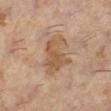<record>
  <biopsy_status>not biopsied; imaged during a skin examination</biopsy_status>
  <lesion_size>
    <long_diameter_mm_approx>5.5</long_diameter_mm_approx>
  </lesion_size>
  <site>left lower leg</site>
  <automated_metrics>
    <area_mm2_approx>13.0</area_mm2_approx>
    <eccentricity>0.8</eccentricity>
    <shape_asymmetry>0.4</shape_asymmetry>
    <color_variation_0_10>2.5</color_variation_0_10>
    <peripheral_color_asymmetry>1.0</peripheral_color_asymmetry>
  </automated_metrics>
  <lighting>cross-polarized</lighting>
  <patient>
    <sex>female</sex>
    <age_approx>60</age_approx>
  </patient>
  <image>
    <source>total-body photography crop</source>
    <field_of_view_mm>15</field_of_view_mm>
  </image>
</record>